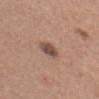workup — no biopsy performed (imaged during a skin exam); lighting — white-light; subject — female, aged approximately 35; anatomic site — the head or neck; acquisition — total-body-photography crop, ~15 mm field of view; diameter — ~2.5 mm (longest diameter).